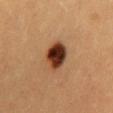Q: Is there a histopathology result?
A: total-body-photography surveillance lesion; no biopsy
Q: How was this image acquired?
A: 15 mm crop, total-body photography
Q: Illumination type?
A: cross-polarized
Q: Where on the body is the lesion?
A: the mid back
Q: What are the patient's age and sex?
A: female, aged around 35
Q: What did automated image analysis measure?
A: a border-irregularity index near 1/10, internal color variation of about 9 on a 0–10 scale, and peripheral color asymmetry of about 2.5; an automated nevus-likeness rating near 100 out of 100
Q: How large is the lesion?
A: ~4 mm (longest diameter)